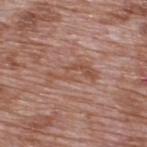This lesion was catalogued during total-body skin photography and was not selected for biopsy. A lesion tile, about 15 mm wide, cut from a 3D total-body photograph. The lesion is on the back. The total-body-photography lesion software estimated two-axis asymmetry of about 0.55. The analysis additionally found a border-irregularity index near 8.5/10, a within-lesion color-variation index near 3/10, and peripheral color asymmetry of about 1. The software also gave a classifier nevus-likeness of about 0/100. A male subject aged around 70. Approximately 5.5 mm at its widest.